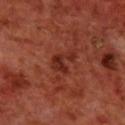Impression: The lesion was photographed on a routine skin check and not biopsied; there is no pathology result. Clinical summary: A close-up tile cropped from a whole-body skin photograph, about 15 mm across. A male patient aged 68–72. Approximately 3 mm at its widest. The lesion-visualizer software estimated an area of roughly 4.5 mm², a shape eccentricity near 0.7, and a shape-asymmetry score of about 0.6 (0 = symmetric). The software also gave a mean CIELAB color near L≈28 a*≈28 b*≈28 and a normalized border contrast of about 8. The software also gave an automated nevus-likeness rating near 70 out of 100 and a lesion-detection confidence of about 100/100. Located on the upper back.